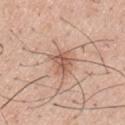Part of a total-body skin-imaging series; this lesion was reviewed on a skin check and was not flagged for biopsy.
A male subject in their 30s.
A 15 mm close-up tile from a total-body photography series done for melanoma screening.
The lesion is located on the upper back.
The lesion's longest dimension is about 3 mm.
The tile uses white-light illumination.